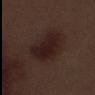Q: Was this lesion biopsied?
A: catalogued during a skin exam; not biopsied
Q: What did automated image analysis measure?
A: a footprint of about 16 mm², a shape eccentricity near 0.75, and a symmetry-axis asymmetry near 0.3; a border-irregularity index near 3/10, a within-lesion color-variation index near 3/10, and a peripheral color-asymmetry measure near 1; a classifier nevus-likeness of about 35/100 and lesion-presence confidence of about 100/100
Q: What lighting was used for the tile?
A: white-light
Q: What kind of image is this?
A: ~15 mm crop, total-body skin-cancer survey
Q: Lesion location?
A: the left thigh
Q: How large is the lesion?
A: about 5.5 mm
Q: What are the patient's age and sex?
A: male, approximately 70 years of age